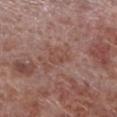<record>
  <biopsy_status>not biopsied; imaged during a skin examination</biopsy_status>
  <image>
    <source>total-body photography crop</source>
    <field_of_view_mm>15</field_of_view_mm>
  </image>
  <patient>
    <sex>male</sex>
    <age_approx>55</age_approx>
  </patient>
  <site>left lower leg</site>
  <lesion_size>
    <long_diameter_mm_approx>3.0</long_diameter_mm_approx>
  </lesion_size>
  <lighting>white-light</lighting>
</record>